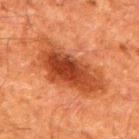Recorded during total-body skin imaging; not selected for excision or biopsy.
A 15 mm close-up extracted from a 3D total-body photography capture.
The tile uses cross-polarized illumination.
The total-body-photography lesion software estimated an area of roughly 29 mm², an outline eccentricity of about 0.9 (0 = round, 1 = elongated), and a symmetry-axis asymmetry near 0.3. The software also gave a nevus-likeness score of about 80/100.
The lesion is on the right upper arm.
The lesion's longest dimension is about 9.5 mm.
A male patient, in their 60s.